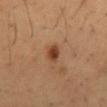Imaged with cross-polarized lighting.
The lesion's longest dimension is about 2.5 mm.
A close-up tile cropped from a whole-body skin photograph, about 15 mm across.
A male subject, roughly 50 years of age.
Located on the abdomen.